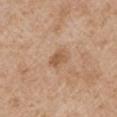Q: Is there a histopathology result?
A: no biopsy performed (imaged during a skin exam)
Q: What did automated image analysis measure?
A: a shape-asymmetry score of about 0.2 (0 = symmetric); a nevus-likeness score of about 20/100 and a lesion-detection confidence of about 100/100
Q: What kind of image is this?
A: ~15 mm tile from a whole-body skin photo
Q: Who is the patient?
A: male, in their mid-60s
Q: What is the anatomic site?
A: the left upper arm
Q: What lighting was used for the tile?
A: white-light
Q: Lesion size?
A: ≈2.5 mm